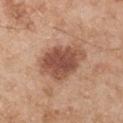{
  "patient": {
    "sex": "male",
    "age_approx": 55
  },
  "image": {
    "source": "total-body photography crop",
    "field_of_view_mm": 15
  },
  "site": "left upper arm"
}